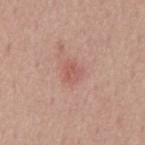Part of a total-body skin-imaging series; this lesion was reviewed on a skin check and was not flagged for biopsy. A male patient aged 53–57. Automated tile analysis of the lesion measured a footprint of about 4.5 mm², a shape eccentricity near 0.55, and a symmetry-axis asymmetry near 0.3. The analysis additionally found border irregularity of about 2.5 on a 0–10 scale, internal color variation of about 2 on a 0–10 scale, and radial color variation of about 0.5. The software also gave lesion-presence confidence of about 100/100. Cropped from a total-body skin-imaging series; the visible field is about 15 mm. Measured at roughly 2.5 mm in maximum diameter. The lesion is on the chest.